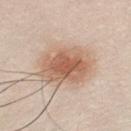Clinical impression: The lesion was photographed on a routine skin check and not biopsied; there is no pathology result. Clinical summary: Automated tile analysis of the lesion measured an area of roughly 24 mm² and a symmetry-axis asymmetry near 0.1. It also reported a border-irregularity rating of about 1.5/10 and a peripheral color-asymmetry measure near 1.5. The analysis additionally found a detector confidence of about 100 out of 100 that the crop contains a lesion. A male patient in their mid- to late 30s. The lesion is located on the chest. A region of skin cropped from a whole-body photographic capture, roughly 15 mm wide. This is a white-light tile.